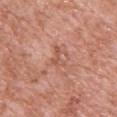{
  "biopsy_status": "not biopsied; imaged during a skin examination",
  "patient": {
    "sex": "male",
    "age_approx": 60
  },
  "lighting": "white-light",
  "lesion_size": {
    "long_diameter_mm_approx": 3.0
  },
  "site": "upper back",
  "image": {
    "source": "total-body photography crop",
    "field_of_view_mm": 15
  }
}